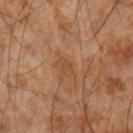| field | value |
|---|---|
| biopsy status | imaged on a skin check; not biopsied |